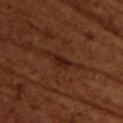follow-up = total-body-photography surveillance lesion; no biopsy | subject = female, about 55 years old | site = the upper back | image source = 15 mm crop, total-body photography.